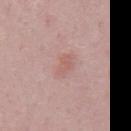{
  "biopsy_status": "not biopsied; imaged during a skin examination",
  "lesion_size": {
    "long_diameter_mm_approx": 3.0
  },
  "lighting": "white-light",
  "image": {
    "source": "total-body photography crop",
    "field_of_view_mm": 15
  },
  "site": "mid back",
  "patient": {
    "sex": "male",
    "age_approx": 50
  }
}